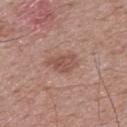follow-up = imaged on a skin check; not biopsied
acquisition = ~15 mm crop, total-body skin-cancer survey
subject = male, aged around 70
automated metrics = an area of roughly 5.5 mm² and a symmetry-axis asymmetry near 0.3; roughly 9 lightness units darker than nearby skin
anatomic site = the back
diameter = ~3.5 mm (longest diameter)
illumination = white-light illumination